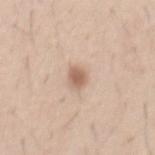– follow-up: catalogued during a skin exam; not biopsied
– image source: ~15 mm tile from a whole-body skin photo
– anatomic site: the mid back
– subject: male, aged approximately 35